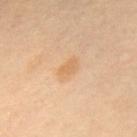Assessment:
The lesion was tiled from a total-body skin photograph and was not biopsied.
Background:
The patient is a female roughly 50 years of age. Located on the upper back. This image is a 15 mm lesion crop taken from a total-body photograph.